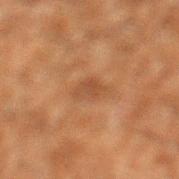Impression: The lesion was tiled from a total-body skin photograph and was not biopsied. Background: A 15 mm close-up tile from a total-body photography series done for melanoma screening. Captured under cross-polarized illumination. A male subject aged approximately 60. From the left lower leg.